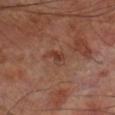This image is a 15 mm lesion crop taken from a total-body photograph.
The lesion is located on the leg.
A male subject aged 68 to 72.